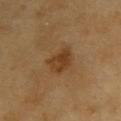Captured during whole-body skin photography for melanoma surveillance; the lesion was not biopsied. A male patient, aged 58 to 62. Measured at roughly 3 mm in maximum diameter. A region of skin cropped from a whole-body photographic capture, roughly 15 mm wide. The lesion is on the upper back. The tile uses cross-polarized illumination.